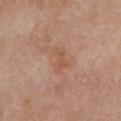Background:
A female subject about 50 years old. Located on the chest. Cropped from a total-body skin-imaging series; the visible field is about 15 mm.